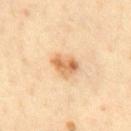Recorded during total-body skin imaging; not selected for excision or biopsy.
Longest diameter approximately 3.5 mm.
Located on the front of the torso.
The patient is a male aged 38–42.
A 15 mm close-up tile from a total-body photography series done for melanoma screening.
This is a cross-polarized tile.
The total-body-photography lesion software estimated roughly 11 lightness units darker than nearby skin and a lesion-to-skin contrast of about 8 (normalized; higher = more distinct). And it measured a border-irregularity index near 2.5/10, a color-variation rating of about 6/10, and peripheral color asymmetry of about 2.